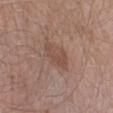On the right lower leg.
Longest diameter approximately 3.5 mm.
A male subject aged 43–47.
Cropped from a whole-body photographic skin survey; the tile spans about 15 mm.
This is a white-light tile.